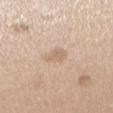Impression: No biopsy was performed on this lesion — it was imaged during a full skin examination and was not determined to be concerning. Acquisition and patient details: A close-up tile cropped from a whole-body skin photograph, about 15 mm across. The patient is a female in their 40s. Located on the arm.